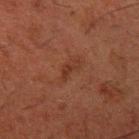Case summary:
- follow-up: total-body-photography surveillance lesion; no biopsy
- body site: the left upper arm
- automated metrics: about 5 CIELAB-L* units darker than the surrounding skin and a lesion-to-skin contrast of about 5.5 (normalized; higher = more distinct); a border-irregularity index near 6/10, internal color variation of about 0 on a 0–10 scale, and a peripheral color-asymmetry measure near 0
- lighting: cross-polarized illumination
- patient: male, approximately 50 years of age
- acquisition: 15 mm crop, total-body photography